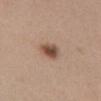Recorded during total-body skin imaging; not selected for excision or biopsy.
A female patient, about 40 years old.
A 15 mm close-up tile from a total-body photography series done for melanoma screening.
The lesion is located on the abdomen.